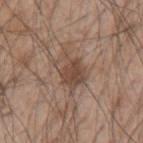biopsy status=catalogued during a skin exam; not biopsied
diameter=about 5 mm
illumination=white-light
image=~15 mm crop, total-body skin-cancer survey
site=the left upper arm
subject=male, aged around 50